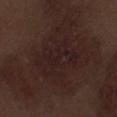The lesion was photographed on a routine skin check and not biopsied; there is no pathology result.
On the leg.
A 15 mm crop from a total-body photograph taken for skin-cancer surveillance.
A male patient, aged 68–72.